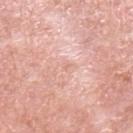notes: total-body-photography surveillance lesion; no biopsy | anatomic site: the left upper arm | imaging modality: ~15 mm tile from a whole-body skin photo | subject: male, in their 80s | size: ~1 mm (longest diameter) | tile lighting: white-light.